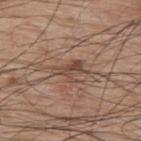Notes:
- follow-up — catalogued during a skin exam; not biopsied
- patient — male, in their 70s
- site — the back
- image — 15 mm crop, total-body photography